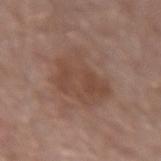| feature | finding |
|---|---|
| notes | imaged on a skin check; not biopsied |
| location | the left forearm |
| lesion diameter | ≈6 mm |
| subject | male, in their mid- to late 50s |
| image source | 15 mm crop, total-body photography |